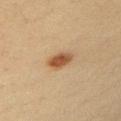Assessment:
Captured during whole-body skin photography for melanoma surveillance; the lesion was not biopsied.
Background:
Located on the left upper arm. A female patient, about 40 years old. This image is a 15 mm lesion crop taken from a total-body photograph.